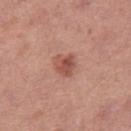follow-up: total-body-photography surveillance lesion; no biopsy | lesion size: about 2.5 mm | TBP lesion metrics: an area of roughly 5 mm² and a shape eccentricity near 0.45; a normalized lesion–skin contrast near 7.5; a border-irregularity index near 2.5/10, internal color variation of about 3.5 on a 0–10 scale, and peripheral color asymmetry of about 1 | image: ~15 mm crop, total-body skin-cancer survey | site: the right thigh | subject: female, aged 38–42.